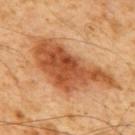Q: Was a biopsy performed?
A: total-body-photography surveillance lesion; no biopsy
Q: What is the lesion's diameter?
A: about 10.5 mm
Q: What are the patient's age and sex?
A: male, in their 60s
Q: Lesion location?
A: the mid back
Q: Automated lesion metrics?
A: an eccentricity of roughly 0.9 and a symmetry-axis asymmetry near 0.45; border irregularity of about 5.5 on a 0–10 scale and radial color variation of about 1.5; an automated nevus-likeness rating near 90 out of 100 and lesion-presence confidence of about 100/100
Q: What lighting was used for the tile?
A: cross-polarized
Q: How was this image acquired?
A: ~15 mm crop, total-body skin-cancer survey